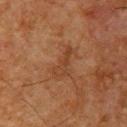follow-up: total-body-photography surveillance lesion; no biopsy
TBP lesion metrics: an eccentricity of roughly 0.95; an average lesion color of about L≈32 a*≈19 b*≈28 (CIELAB) and about 6 CIELAB-L* units darker than the surrounding skin; border irregularity of about 6.5 on a 0–10 scale, a within-lesion color-variation index near 0.5/10, and a peripheral color-asymmetry measure near 0; an automated nevus-likeness rating near 0 out of 100 and a detector confidence of about 100 out of 100 that the crop contains a lesion
anatomic site: the right upper arm
imaging modality: 15 mm crop, total-body photography
illumination: cross-polarized
subject: male, about 65 years old
lesion diameter: about 3.5 mm